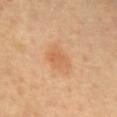Case summary:
• follow-up · no biopsy performed (imaged during a skin exam)
• subject · male, about 65 years old
• location · the mid back
• lighting · cross-polarized illumination
• image · ~15 mm crop, total-body skin-cancer survey
• diameter · about 4.5 mm
• image-analysis metrics · a mean CIELAB color near L≈63 a*≈22 b*≈38, a lesion–skin lightness drop of about 7, and a normalized lesion–skin contrast near 5; a nevus-likeness score of about 45/100 and a detector confidence of about 100 out of 100 that the crop contains a lesion